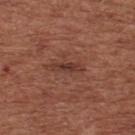Findings:
* notes — no biopsy performed (imaged during a skin exam)
* site — the back
* size — about 3.5 mm
* illumination — white-light illumination
* image — ~15 mm tile from a whole-body skin photo
* subject — male, in their mid- to late 60s
* automated metrics — a footprint of about 3.5 mm² and an outline eccentricity of about 0.95 (0 = round, 1 = elongated); a classifier nevus-likeness of about 10/100 and lesion-presence confidence of about 100/100